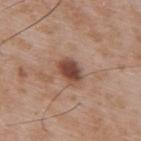This lesion was catalogued during total-body skin photography and was not selected for biopsy. About 3 mm across. Cropped from a whole-body photographic skin survey; the tile spans about 15 mm. On the upper back. Automated image analysis of the tile measured a lesion area of about 6 mm², a shape eccentricity near 0.7, and a symmetry-axis asymmetry near 0.2. And it measured a mean CIELAB color near L≈46 a*≈21 b*≈26, about 14 CIELAB-L* units darker than the surrounding skin, and a normalized border contrast of about 10.5. It also reported a border-irregularity index near 2/10 and a within-lesion color-variation index near 3.5/10. The analysis additionally found lesion-presence confidence of about 100/100. The subject is a male roughly 50 years of age.